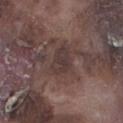The tile uses white-light illumination. From the left lower leg. The patient is a male in their mid-70s. Measured at roughly 3.5 mm in maximum diameter. A 15 mm close-up extracted from a 3D total-body photography capture.